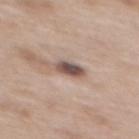This lesion was catalogued during total-body skin photography and was not selected for biopsy.
A 15 mm close-up extracted from a 3D total-body photography capture.
A female patient, approximately 40 years of age.
The lesion is located on the mid back.